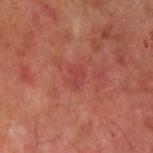This lesion was catalogued during total-body skin photography and was not selected for biopsy. A male patient, aged 63–67. From the left upper arm. A roughly 15 mm field-of-view crop from a total-body skin photograph. Measured at roughly 2.5 mm in maximum diameter. Automated image analysis of the tile measured a lesion color around L≈43 a*≈33 b*≈25 in CIELAB and a normalized border contrast of about 4.5.